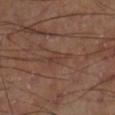follow-up: total-body-photography surveillance lesion; no biopsy | anatomic site: the right lower leg | lesion size: ≈3 mm | image source: ~15 mm crop, total-body skin-cancer survey.